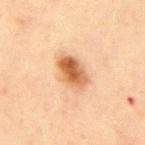Impression: The lesion was tiled from a total-body skin photograph and was not biopsied. Acquisition and patient details: The lesion is located on the mid back. Imaged with cross-polarized lighting. A close-up tile cropped from a whole-body skin photograph, about 15 mm across. Automated image analysis of the tile measured an average lesion color of about L≈54 a*≈21 b*≈35 (CIELAB), about 14 CIELAB-L* units darker than the surrounding skin, and a lesion-to-skin contrast of about 9.5 (normalized; higher = more distinct). And it measured a peripheral color-asymmetry measure near 2.5. Measured at roughly 4.5 mm in maximum diameter. The patient is a male aged 43–47.